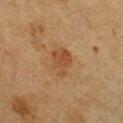follow-up: no biopsy performed (imaged during a skin exam); imaging modality: 15 mm crop, total-body photography; anatomic site: the chest; patient: female, approximately 40 years of age.